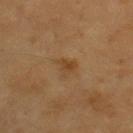Findings:
* notes — catalogued during a skin exam; not biopsied
* patient — male, roughly 60 years of age
* tile lighting — cross-polarized
* automated lesion analysis — a lesion color around L≈43 a*≈18 b*≈35 in CIELAB and a lesion–skin lightness drop of about 7; a border-irregularity rating of about 4/10 and peripheral color asymmetry of about 0.5; a nevus-likeness score of about 30/100
* imaging modality — 15 mm crop, total-body photography
* location — the upper back